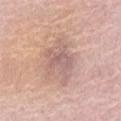  biopsy_status: not biopsied; imaged during a skin examination
  patient:
    sex: female
    age_approx: 60
  site: abdomen
  lighting: white-light
  image:
    source: total-body photography crop
    field_of_view_mm: 15
  automated_metrics:
    area_mm2_approx: 9.5
    eccentricity: 0.65
    cielab_L: 61
    cielab_a: 20
    cielab_b: 22
    vs_skin_darker_L: 8.0
    vs_skin_contrast_norm: 5.5